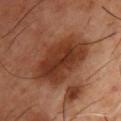Part of a total-body skin-imaging series; this lesion was reviewed on a skin check and was not flagged for biopsy. Automated image analysis of the tile measured a footprint of about 29 mm², an eccentricity of roughly 0.8, and two-axis asymmetry of about 0.25. A 15 mm close-up tile from a total-body photography series done for melanoma screening. Located on the chest. A male patient, aged approximately 55. Imaged with cross-polarized lighting.